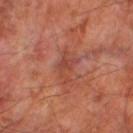image: ~15 mm crop, total-body skin-cancer survey | patient: male, about 70 years old | lesion diameter: ≈4 mm | illumination: cross-polarized illumination | automated metrics: an average lesion color of about L≈44 a*≈28 b*≈29 (CIELAB), about 7 CIELAB-L* units darker than the surrounding skin, and a lesion-to-skin contrast of about 5.5 (normalized; higher = more distinct); a within-lesion color-variation index near 1.5/10 and peripheral color asymmetry of about 0.5; a classifier nevus-likeness of about 0/100 | anatomic site: the right thigh.